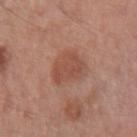{"biopsy_status": "not biopsied; imaged during a skin examination", "patient": {"sex": "female", "age_approx": 50}, "lighting": "white-light", "image": {"source": "total-body photography crop", "field_of_view_mm": 15}, "lesion_size": {"long_diameter_mm_approx": 4.0}, "automated_metrics": {"area_mm2_approx": 9.0, "eccentricity": 0.75, "shape_asymmetry": 0.35, "cielab_L": 49, "cielab_a": 24, "cielab_b": 29, "vs_skin_contrast_norm": 6.0, "nevus_likeness_0_100": 15, "lesion_detection_confidence_0_100": 100}, "site": "right forearm"}